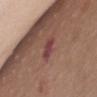Q: What are the patient's age and sex?
A: female, in their mid- to late 60s
Q: How was this image acquired?
A: ~15 mm crop, total-body skin-cancer survey
Q: How was the tile lit?
A: white-light illumination
Q: Lesion location?
A: the front of the torso